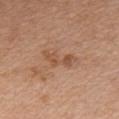The lesion was photographed on a routine skin check and not biopsied; there is no pathology result.
Cropped from a whole-body photographic skin survey; the tile spans about 15 mm.
Captured under white-light illumination.
The recorded lesion diameter is about 4.5 mm.
An algorithmic analysis of the crop reported a lesion–skin lightness drop of about 8. The software also gave a classifier nevus-likeness of about 0/100.
Located on the chest.
A male patient roughly 65 years of age.